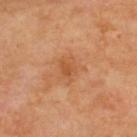The lesion was tiled from a total-body skin photograph and was not biopsied.
A region of skin cropped from a whole-body photographic capture, roughly 15 mm wide.
The lesion's longest dimension is about 2.5 mm.
A male patient in their 70s.
From the upper back.
Imaged with cross-polarized lighting.